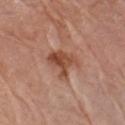Q: Was a biopsy performed?
A: catalogued during a skin exam; not biopsied
Q: Who is the patient?
A: male, roughly 80 years of age
Q: How was this image acquired?
A: total-body-photography crop, ~15 mm field of view
Q: Lesion location?
A: the left forearm
Q: How large is the lesion?
A: ≈4 mm